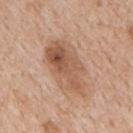site: the mid back | subject: male, about 65 years old | image source: ~15 mm crop, total-body skin-cancer survey | tile lighting: white-light illumination.